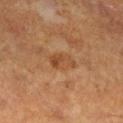workup: catalogued during a skin exam; not biopsied | image: ~15 mm tile from a whole-body skin photo | subject: male, about 60 years old | tile lighting: cross-polarized illumination | lesion diameter: ~3.5 mm (longest diameter) | location: the right lower leg.